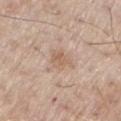Assessment:
No biopsy was performed on this lesion — it was imaged during a full skin examination and was not determined to be concerning.